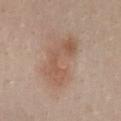| feature | finding |
|---|---|
| subject | female, aged approximately 25 |
| image | ~15 mm crop, total-body skin-cancer survey |
| location | the chest |
| automated lesion analysis | a mean CIELAB color near L≈55 a*≈19 b*≈28, about 8 CIELAB-L* units darker than the surrounding skin, and a lesion-to-skin contrast of about 6 (normalized; higher = more distinct); an automated nevus-likeness rating near 5 out of 100 and a detector confidence of about 100 out of 100 that the crop contains a lesion |
| lesion size | about 6.5 mm |